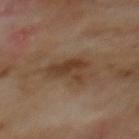Assessment: Captured during whole-body skin photography for melanoma surveillance; the lesion was not biopsied. Clinical summary: Longest diameter approximately 4.5 mm. An algorithmic analysis of the crop reported a detector confidence of about 100 out of 100 that the crop contains a lesion. Imaged with cross-polarized lighting. From the mid back. A male patient, aged 68 to 72. A region of skin cropped from a whole-body photographic capture, roughly 15 mm wide.